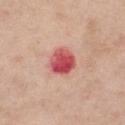biopsy_status: not biopsied; imaged during a skin examination
site: right thigh
image:
  source: total-body photography crop
  field_of_view_mm: 15
lighting: white-light
lesion_size:
  long_diameter_mm_approx: 3.5
automated_metrics:
  border_irregularity_0_10: 1.5
  color_variation_0_10: 7.0
patient:
  sex: female
  age_approx: 55